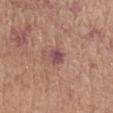This lesion was catalogued during total-body skin photography and was not selected for biopsy.
Located on the left forearm.
Cropped from a whole-body photographic skin survey; the tile spans about 15 mm.
Automated tile analysis of the lesion measured a border-irregularity index near 3/10 and a peripheral color-asymmetry measure near 1.
This is a white-light tile.
The subject is a female in their mid- to late 60s.
The lesion's longest dimension is about 2.5 mm.